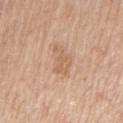workup = total-body-photography surveillance lesion; no biopsy
lesion diameter = ~4.5 mm (longest diameter)
imaging modality = ~15 mm crop, total-body skin-cancer survey
location = the left upper arm
subject = female, aged 68–72
image-analysis metrics = a lesion area of about 6.5 mm² and an eccentricity of roughly 0.85; a border-irregularity rating of about 4.5/10, a within-lesion color-variation index near 2/10, and radial color variation of about 1
tile lighting = white-light illumination